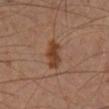Clinical impression:
The lesion was photographed on a routine skin check and not biopsied; there is no pathology result.
Acquisition and patient details:
The patient is a male in their mid- to late 60s. On the right lower leg. A lesion tile, about 15 mm wide, cut from a 3D total-body photograph.